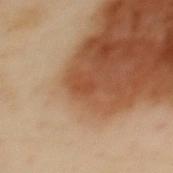Assessment:
The lesion was photographed on a routine skin check and not biopsied; there is no pathology result.
Background:
Imaged with cross-polarized lighting. From the back. A female subject, in their 60s. This image is a 15 mm lesion crop taken from a total-body photograph. The lesion's longest dimension is about 2 mm.